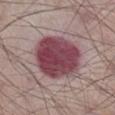biopsy status = catalogued during a skin exam; not biopsied
automated metrics = a lesion color around L≈44 a*≈26 b*≈14 in CIELAB, about 17 CIELAB-L* units darker than the surrounding skin, and a normalized lesion–skin contrast near 12.5; a classifier nevus-likeness of about 100/100 and a detector confidence of about 95 out of 100 that the crop contains a lesion
size = ≈7 mm
acquisition = 15 mm crop, total-body photography
body site = the leg
subject = male, aged 58–62
tile lighting = white-light